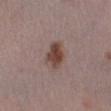Assessment: The lesion was photographed on a routine skin check and not biopsied; there is no pathology result. Image and clinical context: A roughly 15 mm field-of-view crop from a total-body skin photograph. A male patient roughly 55 years of age. The lesion is located on the left lower leg. This is a white-light tile. Measured at roughly 4 mm in maximum diameter.